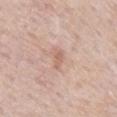follow-up = no biopsy performed (imaged during a skin exam)
subject = male, roughly 85 years of age or older
site = the front of the torso
image = ~15 mm crop, total-body skin-cancer survey